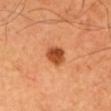The lesion-visualizer software estimated a border-irregularity rating of about 2/10 and radial color variation of about 1.5.
Located on the left upper arm.
A male subject in their 70s.
Approximately 3 mm at its widest.
The tile uses cross-polarized illumination.
Cropped from a total-body skin-imaging series; the visible field is about 15 mm.